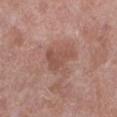Impression:
Captured during whole-body skin photography for melanoma surveillance; the lesion was not biopsied.
Background:
A female patient aged around 50. This image is a 15 mm lesion crop taken from a total-body photograph. This is a white-light tile. On the left lower leg. An algorithmic analysis of the crop reported a lesion area of about 7 mm², an outline eccentricity of about 0.75 (0 = round, 1 = elongated), and two-axis asymmetry of about 0.5. The analysis additionally found about 8 CIELAB-L* units darker than the surrounding skin and a normalized lesion–skin contrast near 6. It also reported a border-irregularity rating of about 5/10 and a color-variation rating of about 2/10. Longest diameter approximately 3.5 mm.